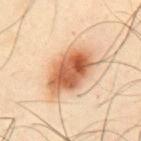Impression:
This lesion was catalogued during total-body skin photography and was not selected for biopsy.
Background:
The lesion is located on the chest. A male patient aged 48–52. The lesion-visualizer software estimated border irregularity of about 2 on a 0–10 scale, internal color variation of about 8 on a 0–10 scale, and a peripheral color-asymmetry measure near 2.5. Cropped from a whole-body photographic skin survey; the tile spans about 15 mm.